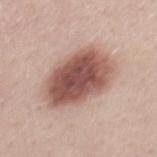acquisition: total-body-photography crop, ~15 mm field of view | anatomic site: the back | subject: male, aged approximately 40.